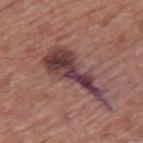Clinical impression: This lesion was catalogued during total-body skin photography and was not selected for biopsy. Image and clinical context: This image is a 15 mm lesion crop taken from a total-body photograph. Located on the mid back. The subject is a male roughly 75 years of age.